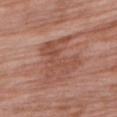This lesion was catalogued during total-body skin photography and was not selected for biopsy. On the right upper arm. A female patient aged 68–72. This is a white-light tile. This image is a 15 mm lesion crop taken from a total-body photograph. Automated tile analysis of the lesion measured a border-irregularity index near 9.5/10 and a color-variation rating of about 3.5/10. And it measured an automated nevus-likeness rating near 0 out of 100 and a detector confidence of about 95 out of 100 that the crop contains a lesion. The lesion's longest dimension is about 5.5 mm.